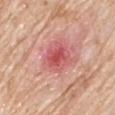The lesion was photographed on a routine skin check and not biopsied; there is no pathology result.
A 15 mm close-up tile from a total-body photography series done for melanoma screening.
The total-body-photography lesion software estimated a classifier nevus-likeness of about 0/100 and a lesion-detection confidence of about 100/100.
The recorded lesion diameter is about 4 mm.
Captured under white-light illumination.
The subject is a male approximately 80 years of age.
On the chest.